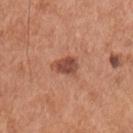  biopsy_status: not biopsied; imaged during a skin examination
  image:
    source: total-body photography crop
    field_of_view_mm: 15
  patient:
    sex: male
    age_approx: 55
  site: chest